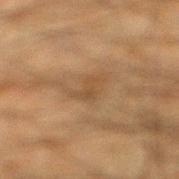Findings:
- notes — imaged on a skin check; not biopsied
- patient — male, roughly 35 years of age
- body site — the right lower leg
- image source — total-body-photography crop, ~15 mm field of view